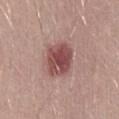Impression:
No biopsy was performed on this lesion — it was imaged during a full skin examination and was not determined to be concerning.
Clinical summary:
The lesion-visualizer software estimated a footprint of about 12 mm². The analysis additionally found a lesion color around L≈48 a*≈24 b*≈20 in CIELAB, roughly 14 lightness units darker than nearby skin, and a normalized lesion–skin contrast near 9.5. The analysis additionally found a classifier nevus-likeness of about 35/100. A male patient approximately 30 years of age. A 15 mm crop from a total-body photograph taken for skin-cancer surveillance. The lesion's longest dimension is about 4 mm.